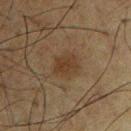Recorded during total-body skin imaging; not selected for excision or biopsy.
Automated tile analysis of the lesion measured an area of roughly 6.5 mm² and a shape-asymmetry score of about 0.2 (0 = symmetric). The analysis additionally found a mean CIELAB color near L≈28 a*≈12 b*≈24.
The subject is a male aged approximately 60.
The lesion is located on the chest.
A close-up tile cropped from a whole-body skin photograph, about 15 mm across.
Approximately 3 mm at its widest.